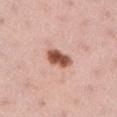<record>
  <biopsy_status>not biopsied; imaged during a skin examination</biopsy_status>
  <lesion_size>
    <long_diameter_mm_approx>3.5</long_diameter_mm_approx>
  </lesion_size>
  <patient>
    <sex>female</sex>
    <age_approx>35</age_approx>
  </patient>
  <lighting>white-light</lighting>
  <image>
    <source>total-body photography crop</source>
    <field_of_view_mm>15</field_of_view_mm>
  </image>
  <site>left lower leg</site>
</record>